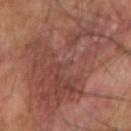This image is a 15 mm lesion crop taken from a total-body photograph.
Located on the right forearm.
A male patient aged 58 to 62.
Approximately 11 mm at its widest.